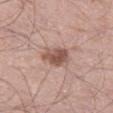Part of a total-body skin-imaging series; this lesion was reviewed on a skin check and was not flagged for biopsy.
On the left thigh.
The subject is a male about 55 years old.
Cropped from a whole-body photographic skin survey; the tile spans about 15 mm.